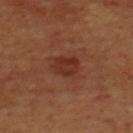Clinical impression:
The lesion was photographed on a routine skin check and not biopsied; there is no pathology result.
Context:
This is a cross-polarized tile. A 15 mm close-up tile from a total-body photography series done for melanoma screening. A male patient aged around 70. The total-body-photography lesion software estimated an eccentricity of roughly 0.55 and a symmetry-axis asymmetry near 0.15. The software also gave a mean CIELAB color near L≈30 a*≈25 b*≈29, roughly 7 lightness units darker than nearby skin, and a normalized lesion–skin contrast near 7. The analysis additionally found an automated nevus-likeness rating near 20 out of 100 and a lesion-detection confidence of about 100/100. The lesion is on the upper back. The lesion's longest dimension is about 3 mm.